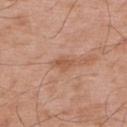Assessment:
Captured during whole-body skin photography for melanoma surveillance; the lesion was not biopsied.
Background:
Located on the upper back. A 15 mm crop from a total-body photograph taken for skin-cancer surveillance. This is a white-light tile. The recorded lesion diameter is about 2.5 mm. A male patient roughly 55 years of age. The lesion-visualizer software estimated an eccentricity of roughly 0.8 and a shape-asymmetry score of about 0.3 (0 = symmetric). And it measured a border-irregularity rating of about 3/10, a within-lesion color-variation index near 1.5/10, and a peripheral color-asymmetry measure near 0.5. The analysis additionally found an automated nevus-likeness rating near 0 out of 100 and a detector confidence of about 100 out of 100 that the crop contains a lesion.